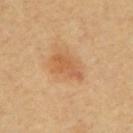Recorded during total-body skin imaging; not selected for excision or biopsy. The patient is aged approximately 60. The lesion is located on the mid back. The lesion's longest dimension is about 4.5 mm. This is a cross-polarized tile. A 15 mm close-up tile from a total-body photography series done for melanoma screening.